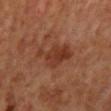Impression: The lesion was tiled from a total-body skin photograph and was not biopsied. Acquisition and patient details: A male subject, aged 58–62. The lesion is on the chest. The total-body-photography lesion software estimated a lesion area of about 11 mm², an eccentricity of roughly 0.8, and a symmetry-axis asymmetry near 0.35. The analysis additionally found an average lesion color of about L≈32 a*≈22 b*≈28 (CIELAB) and a normalized border contrast of about 7. A 15 mm close-up extracted from a 3D total-body photography capture. The tile uses cross-polarized illumination.